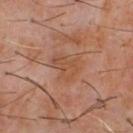Imaged during a routine full-body skin examination; the lesion was not biopsied and no histopathology is available. A male patient, in their 60s. The tile uses cross-polarized illumination. Cropped from a whole-body photographic skin survey; the tile spans about 15 mm. From the chest. Longest diameter approximately 3.5 mm.